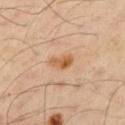This lesion was catalogued during total-body skin photography and was not selected for biopsy. The lesion-visualizer software estimated a lesion area of about 4.5 mm², an eccentricity of roughly 0.85, and two-axis asymmetry of about 0.35. And it measured a color-variation rating of about 5/10 and peripheral color asymmetry of about 1.5. It also reported an automated nevus-likeness rating near 75 out of 100 and lesion-presence confidence of about 100/100. The subject is a male aged 48 to 52. Imaged with cross-polarized lighting. Located on the left upper arm. A region of skin cropped from a whole-body photographic capture, roughly 15 mm wide.